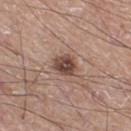Part of a total-body skin-imaging series; this lesion was reviewed on a skin check and was not flagged for biopsy. Located on the right thigh. The tile uses white-light illumination. A roughly 15 mm field-of-view crop from a total-body skin photograph. The lesion's longest dimension is about 3 mm. A male subject, aged 58–62.